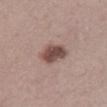Part of a total-body skin-imaging series; this lesion was reviewed on a skin check and was not flagged for biopsy. The lesion's longest dimension is about 3.5 mm. The lesion-visualizer software estimated an area of roughly 8 mm², an eccentricity of roughly 0.6, and two-axis asymmetry of about 0.2. The analysis additionally found an average lesion color of about L≈48 a*≈18 b*≈22 (CIELAB). The analysis additionally found a classifier nevus-likeness of about 75/100. The patient is a female in their 40s. Located on the back. A 15 mm close-up tile from a total-body photography series done for melanoma screening.